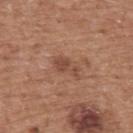• follow-up — total-body-photography surveillance lesion; no biopsy
• acquisition — ~15 mm crop, total-body skin-cancer survey
• site — the upper back
• patient — male, aged 73 to 77
• diameter — ~3.5 mm (longest diameter)
• lighting — white-light